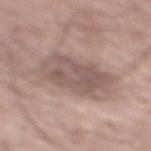{"biopsy_status": "not biopsied; imaged during a skin examination", "automated_metrics": {"area_mm2_approx": 21.0, "eccentricity": 0.8, "shape_asymmetry": 0.2, "border_irregularity_0_10": 2.5, "color_variation_0_10": 3.5, "peripheral_color_asymmetry": 1.5}, "patient": {"sex": "male", "age_approx": 60}, "site": "mid back", "lighting": "white-light", "image": {"source": "total-body photography crop", "field_of_view_mm": 15}}